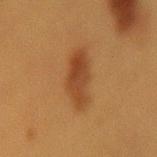follow-up = imaged on a skin check; not biopsied